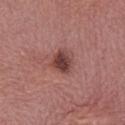notes: total-body-photography surveillance lesion; no biopsy
body site: the chest
diameter: ≈3 mm
patient: female, aged around 65
lighting: white-light illumination
image source: ~15 mm crop, total-body skin-cancer survey